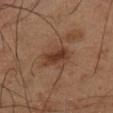Case summary:
* workup: no biopsy performed (imaged during a skin exam)
* lesion size: ~3 mm (longest diameter)
* automated lesion analysis: an average lesion color of about L≈37 a*≈20 b*≈28 (CIELAB), about 9 CIELAB-L* units darker than the surrounding skin, and a normalized border contrast of about 8; an automated nevus-likeness rating near 70 out of 100
* lighting: cross-polarized
* image source: total-body-photography crop, ~15 mm field of view
* site: the leg
* patient: male, aged 58–62